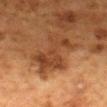{"biopsy_status": "not biopsied; imaged during a skin examination", "patient": {"sex": "female", "age_approx": 50}, "site": "mid back", "image": {"source": "total-body photography crop", "field_of_view_mm": 15}}